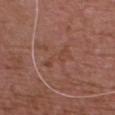notes: imaged on a skin check; not biopsied
TBP lesion metrics: about 5 CIELAB-L* units darker than the surrounding skin and a normalized border contrast of about 4.5; border irregularity of about 6 on a 0–10 scale and a peripheral color-asymmetry measure near 0
anatomic site: the front of the torso
image source: 15 mm crop, total-body photography
subject: male, about 75 years old